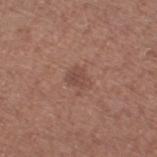  biopsy_status: not biopsied; imaged during a skin examination
  image:
    source: total-body photography crop
    field_of_view_mm: 15
  patient:
    sex: female
    age_approx: 50
  site: right thigh
  automated_metrics:
    border_irregularity_0_10: 3.5
    color_variation_0_10: 2.0
    peripheral_color_asymmetry: 1.0
  lesion_size:
    long_diameter_mm_approx: 2.5
  lighting: white-light